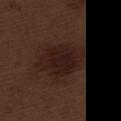No biopsy was performed on this lesion — it was imaged during a full skin examination and was not determined to be concerning. On the left thigh. Longest diameter approximately 6.5 mm. A male patient aged 68 to 72. Imaged with white-light lighting. The total-body-photography lesion software estimated a footprint of about 21 mm², a shape eccentricity near 0.7, and a symmetry-axis asymmetry near 0.2. It also reported a classifier nevus-likeness of about 75/100 and a detector confidence of about 100 out of 100 that the crop contains a lesion. A 15 mm close-up tile from a total-body photography series done for melanoma screening.